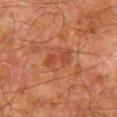Findings:
- follow-up: catalogued during a skin exam; not biopsied
- location: the left thigh
- automated metrics: a nevus-likeness score of about 0/100 and a lesion-detection confidence of about 100/100
- acquisition: 15 mm crop, total-body photography
- subject: male, approximately 80 years of age